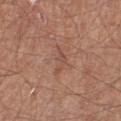Assessment: No biopsy was performed on this lesion — it was imaged during a full skin examination and was not determined to be concerning. Background: Longest diameter approximately 3.5 mm. Located on the right lower leg. This is a white-light tile. A 15 mm close-up tile from a total-body photography series done for melanoma screening. The patient is a male aged 63–67.